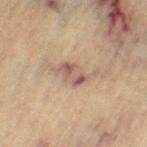| field | value |
|---|---|
| workup | no biopsy performed (imaged during a skin exam) |